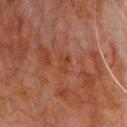This image is a 15 mm lesion crop taken from a total-body photograph.
A male patient aged around 80.
From the chest.